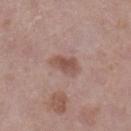workup = imaged on a skin check; not biopsied
image-analysis metrics = a lesion area of about 6 mm², an eccentricity of roughly 0.8, and two-axis asymmetry of about 0.25; a mean CIELAB color near L≈52 a*≈19 b*≈24, roughly 9 lightness units darker than nearby skin, and a normalized border contrast of about 7; border irregularity of about 2.5 on a 0–10 scale, a color-variation rating of about 3/10, and radial color variation of about 1; a nevus-likeness score of about 35/100 and lesion-presence confidence of about 100/100
tile lighting = white-light illumination
acquisition = 15 mm crop, total-body photography
lesion diameter = ~3.5 mm (longest diameter)
patient = male, aged 68 to 72
location = the leg